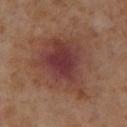follow-up: catalogued during a skin exam; not biopsied | subject: female, in their mid-50s | body site: the right lower leg | image: 15 mm crop, total-body photography | size: ~7.5 mm (longest diameter) | illumination: cross-polarized illumination.